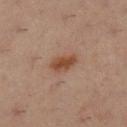Clinical impression: Imaged during a routine full-body skin examination; the lesion was not biopsied and no histopathology is available. Context: On the left lower leg. Approximately 3.5 mm at its widest. A female patient aged 33–37. A close-up tile cropped from a whole-body skin photograph, about 15 mm across.